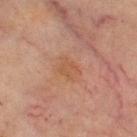Case summary:
• follow-up: no biopsy performed (imaged during a skin exam)
• TBP lesion metrics: a footprint of about 4 mm² and two-axis asymmetry of about 0.45; an average lesion color of about L≈46 a*≈20 b*≈30 (CIELAB) and a normalized lesion–skin contrast near 5; internal color variation of about 1.5 on a 0–10 scale and a peripheral color-asymmetry measure near 0.5; an automated nevus-likeness rating near 0 out of 100 and a detector confidence of about 100 out of 100 that the crop contains a lesion
• acquisition: ~15 mm crop, total-body skin-cancer survey
• size: about 2.5 mm
• subject: female, roughly 70 years of age
• illumination: cross-polarized
• anatomic site: the leg